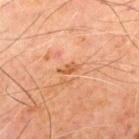image:
  source: total-body photography crop
  field_of_view_mm: 15
patient:
  sex: male
  age_approx: 70
site: chest
lighting: cross-polarized